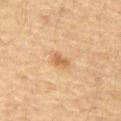biopsy status: total-body-photography surveillance lesion; no biopsy
anatomic site: the abdomen
image-analysis metrics: a lesion color around L≈52 a*≈17 b*≈34 in CIELAB, roughly 8 lightness units darker than nearby skin, and a lesion-to-skin contrast of about 6.5 (normalized; higher = more distinct); a within-lesion color-variation index near 2/10 and radial color variation of about 0.5; an automated nevus-likeness rating near 45 out of 100 and a detector confidence of about 100 out of 100 that the crop contains a lesion
imaging modality: ~15 mm tile from a whole-body skin photo
illumination: cross-polarized illumination
subject: male, approximately 65 years of age